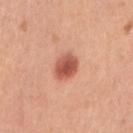No biopsy was performed on this lesion — it was imaged during a full skin examination and was not determined to be concerning. A female subject roughly 60 years of age. A close-up tile cropped from a whole-body skin photograph, about 15 mm across. Longest diameter approximately 3 mm. From the right upper arm. An algorithmic analysis of the crop reported an automated nevus-likeness rating near 100 out of 100 and a lesion-detection confidence of about 100/100.